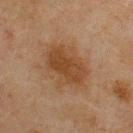{"biopsy_status": "not biopsied; imaged during a skin examination", "image": {"source": "total-body photography crop", "field_of_view_mm": 15}, "site": "back", "patient": {"sex": "male", "age_approx": 45}, "automated_metrics": {"area_mm2_approx": 17.0, "cielab_L": 36, "cielab_a": 17, "cielab_b": 29, "vs_skin_darker_L": 8.0, "vs_skin_contrast_norm": 7.5, "color_variation_0_10": 2.5, "nevus_likeness_0_100": 65, "lesion_detection_confidence_0_100": 100}, "lesion_size": {"long_diameter_mm_approx": 6.0}, "lighting": "cross-polarized"}